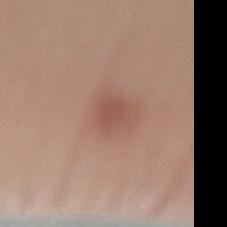anatomic site = the right lower leg | lesion diameter = about 3 mm | lighting = white-light illumination | TBP lesion metrics = an area of roughly 5.5 mm² and a shape eccentricity near 0.45; a border-irregularity rating of about 2.5/10 and a within-lesion color-variation index near 1.5/10 | image source = 15 mm crop, total-body photography | patient = male, approximately 30 years of age.